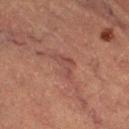biopsy status: no biopsy performed (imaged during a skin exam); body site: the right thigh; illumination: cross-polarized; subject: female, in their 70s; image source: total-body-photography crop, ~15 mm field of view; lesion size: about 3.5 mm.